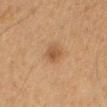workup: catalogued during a skin exam; not biopsied | tile lighting: cross-polarized illumination | image source: total-body-photography crop, ~15 mm field of view | location: the mid back | automated metrics: an area of roughly 4.5 mm² and an outline eccentricity of about 0.55 (0 = round, 1 = elongated); a lesion color around L≈43 a*≈17 b*≈30 in CIELAB; a nevus-likeness score of about 75/100 and lesion-presence confidence of about 100/100 | subject: male, in their mid- to late 60s | lesion size: ~2.5 mm (longest diameter).